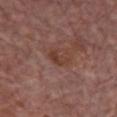The lesion was photographed on a routine skin check and not biopsied; there is no pathology result.
Imaged with white-light lighting.
About 3 mm across.
A 15 mm close-up tile from a total-body photography series done for melanoma screening.
Located on the front of the torso.
A male subject roughly 85 years of age or older.